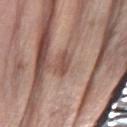Recorded during total-body skin imaging; not selected for excision or biopsy. A close-up tile cropped from a whole-body skin photograph, about 15 mm across. A male patient aged around 70. From the left forearm.